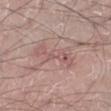follow-up = no biopsy performed (imaged during a skin exam) | lesion size = about 5.5 mm | imaging modality = ~15 mm crop, total-body skin-cancer survey | image-analysis metrics = a classifier nevus-likeness of about 0/100 and a lesion-detection confidence of about 50/100 | anatomic site = the left lower leg | subject = male, in their 30s.